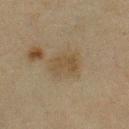Impression:
The lesion was photographed on a routine skin check and not biopsied; there is no pathology result.
Clinical summary:
On the chest. Cropped from a total-body skin-imaging series; the visible field is about 15 mm. An algorithmic analysis of the crop reported an area of roughly 9 mm² and a shape-asymmetry score of about 0.2 (0 = symmetric). The software also gave an average lesion color of about L≈39 a*≈10 b*≈27 (CIELAB) and a normalized lesion–skin contrast near 6. And it measured a border-irregularity index near 2.5/10 and internal color variation of about 2 on a 0–10 scale. The tile uses cross-polarized illumination. A male patient roughly 45 years of age.